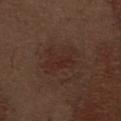Impression:
Part of a total-body skin-imaging series; this lesion was reviewed on a skin check and was not flagged for biopsy.
Acquisition and patient details:
Cropped from a whole-body photographic skin survey; the tile spans about 15 mm. On the abdomen. A male patient, aged 68 to 72. Imaged with white-light lighting.